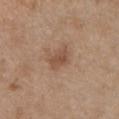biopsy status — total-body-photography surveillance lesion; no biopsy
automated metrics — an outline eccentricity of about 0.75 (0 = round, 1 = elongated) and a symmetry-axis asymmetry near 0.25; a mean CIELAB color near L≈50 a*≈18 b*≈30, a lesion–skin lightness drop of about 9, and a normalized lesion–skin contrast near 6.5; an automated nevus-likeness rating near 35 out of 100
anatomic site — the arm
acquisition — 15 mm crop, total-body photography
lighting — white-light illumination
lesion size — ≈3 mm
patient — female, aged 38–42